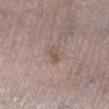workup=no biopsy performed (imaged during a skin exam); automated lesion analysis=an area of roughly 2.5 mm², an eccentricity of roughly 0.9, and two-axis asymmetry of about 0.3; illumination=white-light; subject=male, in their 80s; size=≈2.5 mm; acquisition=~15 mm crop, total-body skin-cancer survey; body site=the left lower leg.